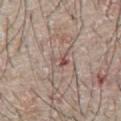Assessment:
Recorded during total-body skin imaging; not selected for excision or biopsy.
Context:
A male subject in their mid-60s. Automated image analysis of the tile measured a mean CIELAB color near L≈54 a*≈17 b*≈22, about 8 CIELAB-L* units darker than the surrounding skin, and a lesion-to-skin contrast of about 5.5 (normalized; higher = more distinct). It also reported a border-irregularity rating of about 5/10, a color-variation rating of about 8.5/10, and radial color variation of about 3.5. From the chest. This is a white-light tile. A region of skin cropped from a whole-body photographic capture, roughly 15 mm wide. The recorded lesion diameter is about 3 mm.